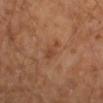workup — imaged on a skin check; not biopsied | patient — male, aged around 60 | lighting — cross-polarized | lesion diameter — about 3 mm | site — the right upper arm | image source — total-body-photography crop, ~15 mm field of view | TBP lesion metrics — an area of roughly 4 mm², an eccentricity of roughly 0.9, and a shape-asymmetry score of about 0.3 (0 = symmetric); a mean CIELAB color near L≈42 a*≈21 b*≈32, a lesion–skin lightness drop of about 6, and a normalized lesion–skin contrast near 5.5; a nevus-likeness score of about 0/100 and a detector confidence of about 100 out of 100 that the crop contains a lesion.